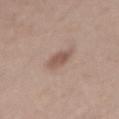This image is a 15 mm lesion crop taken from a total-body photograph. A male patient about 30 years old. The lesion is located on the right upper arm.